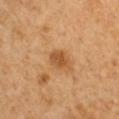Q: Was this lesion biopsied?
A: catalogued during a skin exam; not biopsied
Q: What lighting was used for the tile?
A: cross-polarized
Q: Lesion location?
A: the left upper arm
Q: Who is the patient?
A: male, approximately 50 years of age
Q: What is the imaging modality?
A: ~15 mm crop, total-body skin-cancer survey
Q: What is the lesion's diameter?
A: ~3 mm (longest diameter)